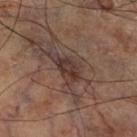Notes:
– follow-up: no biopsy performed (imaged during a skin exam)
– location: the leg
– imaging modality: ~15 mm tile from a whole-body skin photo
– tile lighting: cross-polarized